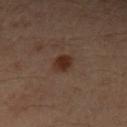This lesion was catalogued during total-body skin photography and was not selected for biopsy. The recorded lesion diameter is about 2.5 mm. A female subject aged 53–57. Captured under cross-polarized illumination. Located on the left forearm. Cropped from a whole-body photographic skin survey; the tile spans about 15 mm.